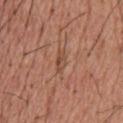The subject is a male in their mid- to late 50s. This is a white-light tile. The lesion's longest dimension is about 2.5 mm. From the head or neck. Cropped from a whole-body photographic skin survey; the tile spans about 15 mm.